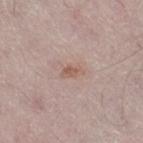{
  "image": {
    "source": "total-body photography crop",
    "field_of_view_mm": 15
  },
  "site": "right thigh",
  "lesion_size": {
    "long_diameter_mm_approx": 2.5
  },
  "patient": {
    "sex": "male",
    "age_approx": 75
  }
}